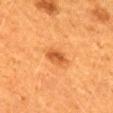Captured during whole-body skin photography for melanoma surveillance; the lesion was not biopsied.